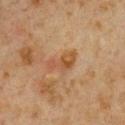The lesion was tiled from a total-body skin photograph and was not biopsied.
The subject is a male about 60 years old.
This is a cross-polarized tile.
A roughly 15 mm field-of-view crop from a total-body skin photograph.
The lesion is located on the chest.